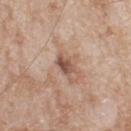<lesion>
<biopsy_status>not biopsied; imaged during a skin examination</biopsy_status>
<image>
  <source>total-body photography crop</source>
  <field_of_view_mm>15</field_of_view_mm>
</image>
<patient>
  <sex>male</sex>
  <age_approx>80</age_approx>
</patient>
<site>back</site>
</lesion>